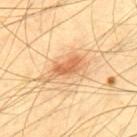Q: Automated lesion metrics?
A: an average lesion color of about L≈67 a*≈23 b*≈41 (CIELAB), about 12 CIELAB-L* units darker than the surrounding skin, and a normalized border contrast of about 7.5; a nevus-likeness score of about 90/100 and a lesion-detection confidence of about 100/100
Q: Illumination type?
A: cross-polarized illumination
Q: Lesion location?
A: the upper back
Q: What kind of image is this?
A: total-body-photography crop, ~15 mm field of view
Q: Lesion size?
A: ≈5 mm
Q: Patient demographics?
A: male, in their mid-60s